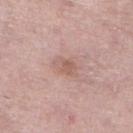notes — no biopsy performed (imaged during a skin exam)
patient — female, aged 58 to 62
illumination — white-light
image — ~15 mm crop, total-body skin-cancer survey
body site — the right lower leg